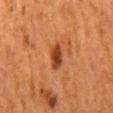Captured during whole-body skin photography for melanoma surveillance; the lesion was not biopsied. The subject is a female aged 48 to 52. The lesion-visualizer software estimated an area of roughly 5 mm², an outline eccentricity of about 0.85 (0 = round, 1 = elongated), and a symmetry-axis asymmetry near 0.3. And it measured an automated nevus-likeness rating near 100 out of 100 and lesion-presence confidence of about 100/100. Imaged with cross-polarized lighting. The lesion is on the mid back. The lesion's longest dimension is about 3.5 mm. A lesion tile, about 15 mm wide, cut from a 3D total-body photograph.